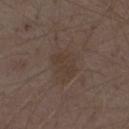Clinical impression: This lesion was catalogued during total-body skin photography and was not selected for biopsy.